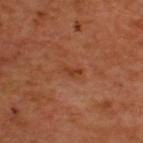Clinical impression:
This lesion was catalogued during total-body skin photography and was not selected for biopsy.
Context:
A male subject, roughly 50 years of age. Longest diameter approximately 3 mm. A 15 mm close-up extracted from a 3D total-body photography capture. The lesion is on the upper back.